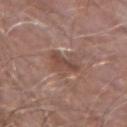Cropped from a total-body skin-imaging series; the visible field is about 15 mm. An algorithmic analysis of the crop reported a border-irregularity rating of about 4.5/10 and peripheral color asymmetry of about 1. And it measured a classifier nevus-likeness of about 0/100 and lesion-presence confidence of about 85/100. The lesion's longest dimension is about 3.5 mm. From the left forearm. A male subject in their mid- to late 60s. Captured under white-light illumination.